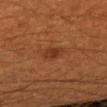Captured during whole-body skin photography for melanoma surveillance; the lesion was not biopsied. The patient is a male aged 58 to 62. Imaged with cross-polarized lighting. The lesion-visualizer software estimated a footprint of about 4.5 mm² and a symmetry-axis asymmetry near 0.2. It also reported a border-irregularity index near 2/10 and a within-lesion color-variation index near 2/10. A 15 mm close-up tile from a total-body photography series done for melanoma screening. Measured at roughly 2.5 mm in maximum diameter. The lesion is located on the right upper arm.